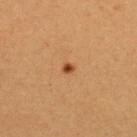image: total-body-photography crop, ~15 mm field of view | body site: the upper back | image-analysis metrics: an eccentricity of roughly 0.55 and a shape-asymmetry score of about 0.25 (0 = symmetric); border irregularity of about 2 on a 0–10 scale and a color-variation rating of about 0/10; lesion-presence confidence of about 100/100 | patient: female, roughly 25 years of age | illumination: cross-polarized | diameter: ~1.5 mm (longest diameter).